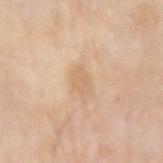Clinical summary: Captured under white-light illumination. From the right upper arm. A male patient approximately 80 years of age. A 15 mm close-up tile from a total-body photography series done for melanoma screening. Automated image analysis of the tile measured a footprint of about 3.5 mm², an outline eccentricity of about 0.75 (0 = round, 1 = elongated), and a shape-asymmetry score of about 0.25 (0 = symmetric). The analysis additionally found border irregularity of about 2 on a 0–10 scale, a color-variation rating of about 1.5/10, and peripheral color asymmetry of about 0.5.